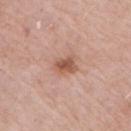Part of a total-body skin-imaging series; this lesion was reviewed on a skin check and was not flagged for biopsy.
Located on the arm.
A 15 mm crop from a total-body photograph taken for skin-cancer surveillance.
The total-body-photography lesion software estimated a lesion color around L≈56 a*≈23 b*≈29 in CIELAB, a lesion–skin lightness drop of about 11, and a normalized border contrast of about 7.5. The analysis additionally found peripheral color asymmetry of about 1. And it measured a nevus-likeness score of about 80/100 and lesion-presence confidence of about 100/100.
A female subject, aged approximately 70.